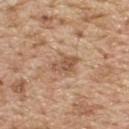Assessment: Part of a total-body skin-imaging series; this lesion was reviewed on a skin check and was not flagged for biopsy. Image and clinical context: This is a white-light tile. The lesion's longest dimension is about 3.5 mm. Cropped from a total-body skin-imaging series; the visible field is about 15 mm. Automated image analysis of the tile measured an area of roughly 6 mm², a shape eccentricity near 0.75, and two-axis asymmetry of about 0.35. A male patient roughly 70 years of age. From the upper back.